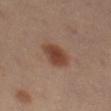A male subject, approximately 60 years of age.
Located on the left thigh.
This image is a 15 mm lesion crop taken from a total-body photograph.